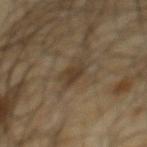The lesion was tiled from a total-body skin photograph and was not biopsied. Automated tile analysis of the lesion measured about 8 CIELAB-L* units darker than the surrounding skin and a normalized lesion–skin contrast near 7.5. The analysis additionally found a border-irregularity rating of about 3.5/10, a within-lesion color-variation index near 1.5/10, and peripheral color asymmetry of about 0.5. A male patient in their mid- to late 60s. Captured under cross-polarized illumination. Longest diameter approximately 3 mm. A lesion tile, about 15 mm wide, cut from a 3D total-body photograph. The lesion is located on the mid back.